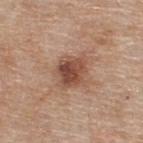workup=total-body-photography surveillance lesion; no biopsy
subject=male, aged 53 to 57
image source=total-body-photography crop, ~15 mm field of view
diameter=~4 mm (longest diameter)
body site=the back
illumination=white-light illumination
image-analysis metrics=an area of roughly 11 mm², an eccentricity of roughly 0.5, and two-axis asymmetry of about 0.25; an automated nevus-likeness rating near 70 out of 100